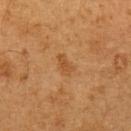Imaged with cross-polarized lighting.
A male patient, roughly 60 years of age.
Longest diameter approximately 2.5 mm.
Located on the left upper arm.
Cropped from a whole-body photographic skin survey; the tile spans about 15 mm.
The total-body-photography lesion software estimated a border-irregularity rating of about 4/10, a within-lesion color-variation index near 1.5/10, and peripheral color asymmetry of about 0.5.